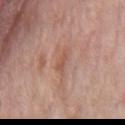workup=catalogued during a skin exam; not biopsied
image source=~15 mm tile from a whole-body skin photo
subject=male, aged approximately 55
tile lighting=white-light illumination
lesion diameter=~2.5 mm (longest diameter)
body site=the chest